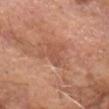Impression: This lesion was catalogued during total-body skin photography and was not selected for biopsy. Acquisition and patient details: The lesion's longest dimension is about 3 mm. A 15 mm close-up tile from a total-body photography series done for melanoma screening. The total-body-photography lesion software estimated a nevus-likeness score of about 0/100 and a detector confidence of about 85 out of 100 that the crop contains a lesion. The lesion is located on the head or neck. A male subject about 85 years old or older. The tile uses white-light illumination.